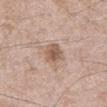<record>
<biopsy_status>not biopsied; imaged during a skin examination</biopsy_status>
<lesion_size>
  <long_diameter_mm_approx>3.5</long_diameter_mm_approx>
</lesion_size>
<image>
  <source>total-body photography crop</source>
  <field_of_view_mm>15</field_of_view_mm>
</image>
<automated_metrics>
  <cielab_L>57</cielab_L>
  <cielab_a>18</cielab_a>
  <cielab_b>28</cielab_b>
  <vs_skin_contrast_norm>7.5</vs_skin_contrast_norm>
</automated_metrics>
<site>chest</site>
<patient>
  <sex>male</sex>
  <age_approx>50</age_approx>
</patient>
</record>